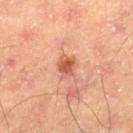<case>
  <biopsy_status>not biopsied; imaged during a skin examination</biopsy_status>
  <patient>
    <sex>male</sex>
    <age_approx>65</age_approx>
  </patient>
  <lesion_size>
    <long_diameter_mm_approx>2.5</long_diameter_mm_approx>
  </lesion_size>
  <site>left thigh</site>
  <image>
    <source>total-body photography crop</source>
    <field_of_view_mm>15</field_of_view_mm>
  </image>
</case>